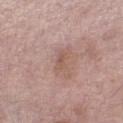{"biopsy_status": "not biopsied; imaged during a skin examination", "patient": {"sex": "female", "age_approx": 75}, "site": "left lower leg", "lesion_size": {"long_diameter_mm_approx": 2.5}, "image": {"source": "total-body photography crop", "field_of_view_mm": 15}}